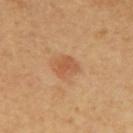Impression:
Captured during whole-body skin photography for melanoma surveillance; the lesion was not biopsied.
Acquisition and patient details:
About 2.5 mm across. A region of skin cropped from a whole-body photographic capture, roughly 15 mm wide. The lesion is located on the upper back. The subject is aged 58–62. Imaged with cross-polarized lighting.